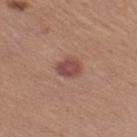This lesion was catalogued during total-body skin photography and was not selected for biopsy.
A 15 mm crop from a total-body photograph taken for skin-cancer surveillance.
A female patient, in their mid- to late 50s.
The lesion is on the right thigh.